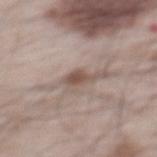No biopsy was performed on this lesion — it was imaged during a full skin examination and was not determined to be concerning. The patient is a male about 65 years old. A lesion tile, about 15 mm wide, cut from a 3D total-body photograph. On the mid back.